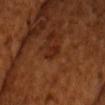follow-up: imaged on a skin check; not biopsied | diameter: about 2.5 mm | patient: male, in their mid- to late 60s | image-analysis metrics: a lesion area of about 2.5 mm², a shape eccentricity near 0.9, and two-axis asymmetry of about 0.4; a lesion color around L≈24 a*≈23 b*≈29 in CIELAB, roughly 7 lightness units darker than nearby skin, and a lesion-to-skin contrast of about 7.5 (normalized; higher = more distinct); an automated nevus-likeness rating near 0 out of 100 and lesion-presence confidence of about 100/100 | anatomic site: the head or neck | lighting: cross-polarized | imaging modality: total-body-photography crop, ~15 mm field of view.